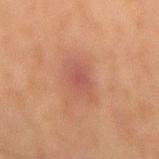Notes:
- biopsy status: imaged on a skin check; not biopsied
- body site: the back
- lesion size: ~4 mm (longest diameter)
- acquisition: total-body-photography crop, ~15 mm field of view
- patient: male, aged around 65
- lighting: cross-polarized